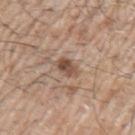Captured during whole-body skin photography for melanoma surveillance; the lesion was not biopsied.
Located on the left upper arm.
A 15 mm close-up tile from a total-body photography series done for melanoma screening.
A male patient, aged 63 to 67.